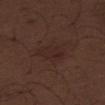Part of a total-body skin-imaging series; this lesion was reviewed on a skin check and was not flagged for biopsy. From the right thigh. A male subject, roughly 70 years of age. A 15 mm close-up tile from a total-body photography series done for melanoma screening.